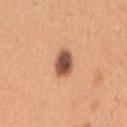Recorded during total-body skin imaging; not selected for excision or biopsy. Measured at roughly 3 mm in maximum diameter. A female patient, aged approximately 30. This is a white-light tile. A lesion tile, about 15 mm wide, cut from a 3D total-body photograph. On the right upper arm.